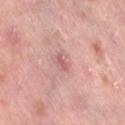Q: Is there a histopathology result?
A: catalogued during a skin exam; not biopsied
Q: What is the imaging modality?
A: 15 mm crop, total-body photography
Q: Patient demographics?
A: female, aged around 50
Q: What did automated image analysis measure?
A: a lesion area of about 2.5 mm², an eccentricity of roughly 0.9, and a shape-asymmetry score of about 0.4 (0 = symmetric); a mean CIELAB color near L≈57 a*≈25 b*≈20 and a lesion–skin lightness drop of about 9
Q: What is the lesion's diameter?
A: ~2.5 mm (longest diameter)
Q: How was the tile lit?
A: cross-polarized illumination
Q: Where on the body is the lesion?
A: the left thigh